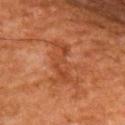Clinical impression: The lesion was tiled from a total-body skin photograph and was not biopsied. Acquisition and patient details: This image is a 15 mm lesion crop taken from a total-body photograph. An algorithmic analysis of the crop reported a lesion color around L≈42 a*≈27 b*≈36 in CIELAB and a lesion–skin lightness drop of about 7. The analysis additionally found a nevus-likeness score of about 0/100 and lesion-presence confidence of about 100/100. The subject is a male aged 58 to 62. On the upper back.